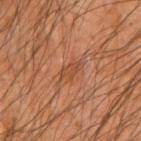Assessment: Recorded during total-body skin imaging; not selected for excision or biopsy.